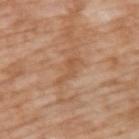Assessment:
No biopsy was performed on this lesion — it was imaged during a full skin examination and was not determined to be concerning.
Clinical summary:
A male subject, aged approximately 60. This image is a 15 mm lesion crop taken from a total-body photograph. The lesion's longest dimension is about 3.5 mm. Located on the upper back.